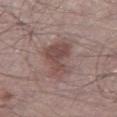- workup: total-body-photography surveillance lesion; no biopsy
- lesion diameter: ~5.5 mm (longest diameter)
- location: the left thigh
- patient: male, about 55 years old
- tile lighting: white-light
- image: 15 mm crop, total-body photography